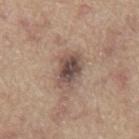Impression:
Part of a total-body skin-imaging series; this lesion was reviewed on a skin check and was not flagged for biopsy.